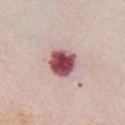Clinical impression: Recorded during total-body skin imaging; not selected for excision or biopsy. Background: A female patient, in their mid- to late 60s. A 15 mm close-up tile from a total-body photography series done for melanoma screening. The lesion is on the chest.